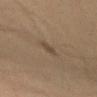Assessment: Imaged during a routine full-body skin examination; the lesion was not biopsied and no histopathology is available. Acquisition and patient details: From the left forearm. A 15 mm close-up tile from a total-body photography series done for melanoma screening. A male patient about 40 years old. An algorithmic analysis of the crop reported a mean CIELAB color near L≈39 a*≈12 b*≈23, a lesion–skin lightness drop of about 6, and a lesion-to-skin contrast of about 5.5 (normalized; higher = more distinct).